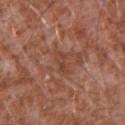An algorithmic analysis of the crop reported a border-irregularity index near 4/10, a within-lesion color-variation index near 0/10, and a peripheral color-asymmetry measure near 0. A region of skin cropped from a whole-body photographic capture, roughly 15 mm wide. This is a white-light tile. From the front of the torso. The subject is a male in their mid- to late 40s. Approximately 3 mm at its widest.